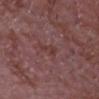Assessment:
Recorded during total-body skin imaging; not selected for excision or biopsy.
Clinical summary:
A roughly 15 mm field-of-view crop from a total-body skin photograph. An algorithmic analysis of the crop reported a footprint of about 2.5 mm² and two-axis asymmetry of about 0.5. And it measured a mean CIELAB color near L≈37 a*≈22 b*≈20. It also reported a within-lesion color-variation index near 0/10 and a peripheral color-asymmetry measure near 0. The software also gave a nevus-likeness score of about 0/100 and lesion-presence confidence of about 95/100. A male subject aged 63–67. On the chest.